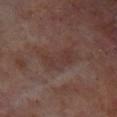Clinical impression: The lesion was photographed on a routine skin check and not biopsied; there is no pathology result. Context: Located on the left lower leg. A male patient aged 68–72. A close-up tile cropped from a whole-body skin photograph, about 15 mm across. This is a cross-polarized tile.